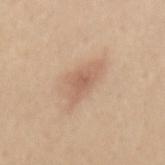Q: Was a biopsy performed?
A: imaged on a skin check; not biopsied
Q: How was this image acquired?
A: total-body-photography crop, ~15 mm field of view
Q: Who is the patient?
A: female, about 35 years old
Q: What lighting was used for the tile?
A: white-light illumination
Q: What is the anatomic site?
A: the mid back